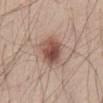<lesion>
  <biopsy_status>not biopsied; imaged during a skin examination</biopsy_status>
  <lesion_size>
    <long_diameter_mm_approx>4.0</long_diameter_mm_approx>
  </lesion_size>
  <image>
    <source>total-body photography crop</source>
    <field_of_view_mm>15</field_of_view_mm>
  </image>
  <patient>
    <sex>male</sex>
    <age_approx>45</age_approx>
  </patient>
  <site>abdomen</site>
  <lighting>white-light</lighting>
</lesion>